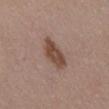{
  "image": {
    "source": "total-body photography crop",
    "field_of_view_mm": 15
  },
  "site": "abdomen",
  "patient": {
    "sex": "female",
    "age_approx": 70
  },
  "lesion_size": {
    "long_diameter_mm_approx": 5.5
  },
  "lighting": "white-light"
}